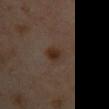Clinical impression: Imaged during a routine full-body skin examination; the lesion was not biopsied and no histopathology is available. Acquisition and patient details: On the chest. A female subject aged around 40. Approximately 2.5 mm at its widest. A close-up tile cropped from a whole-body skin photograph, about 15 mm across. This is a cross-polarized tile.